A female subject, aged 58–62 · this image is a 15 mm lesion crop taken from a total-body photograph · from the left lower leg: 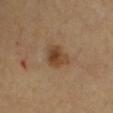<tbp_lesion>
  <diagnosis>
    <histopathology>solar lentigo</histopathology>
    <malignancy>benign</malignancy>
    <taxonomic_path>Benign, Benign epidermal proliferations, Solar lentigo</taxonomic_path>
  </diagnosis>
</tbp_lesion>